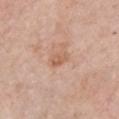{"biopsy_status": "not biopsied; imaged during a skin examination", "image": {"source": "total-body photography crop", "field_of_view_mm": 15}, "automated_metrics": {"cielab_L": 60, "cielab_a": 21, "cielab_b": 32, "vs_skin_contrast_norm": 6.0, "nevus_likeness_0_100": 0, "lesion_detection_confidence_0_100": 100}, "site": "front of the torso", "lighting": "white-light", "patient": {"sex": "female", "age_approx": 65}}